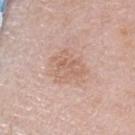<lesion>
<biopsy_status>not biopsied; imaged during a skin examination</biopsy_status>
<image>
  <source>total-body photography crop</source>
  <field_of_view_mm>15</field_of_view_mm>
</image>
<patient>
  <sex>female</sex>
  <age_approx>45</age_approx>
</patient>
<lighting>white-light</lighting>
<site>head or neck</site>
</lesion>